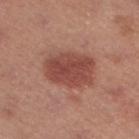• follow-up — imaged on a skin check; not biopsied
• automated lesion analysis — a lesion-to-skin contrast of about 8.5 (normalized; higher = more distinct)
• site — the left lower leg
• patient — female, aged around 40
• size — ~5.5 mm (longest diameter)
• image source — 15 mm crop, total-body photography